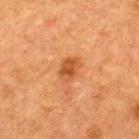This lesion was catalogued during total-body skin photography and was not selected for biopsy.
Located on the upper back.
The recorded lesion diameter is about 3 mm.
Captured under cross-polarized illumination.
A region of skin cropped from a whole-body photographic capture, roughly 15 mm wide.
A male subject aged 48 to 52.